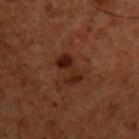notes: total-body-photography surveillance lesion; no biopsy | diameter: about 3.5 mm | imaging modality: 15 mm crop, total-body photography | automated lesion analysis: a shape-asymmetry score of about 0.3 (0 = symmetric); a within-lesion color-variation index near 4/10 and radial color variation of about 1.5; a nevus-likeness score of about 25/100 and a detector confidence of about 100 out of 100 that the crop contains a lesion | location: the upper back | lighting: cross-polarized illumination | patient: male, aged around 60.